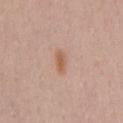workup = no biopsy performed (imaged during a skin exam) | diameter = ~2.5 mm (longest diameter) | body site = the chest | lighting = white-light illumination | subject = male, aged around 35 | image = ~15 mm tile from a whole-body skin photo.